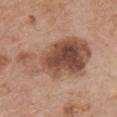| feature | finding |
|---|---|
| location | the chest |
| patient | male, aged 58 to 62 |
| diameter | ≈9.5 mm |
| automated metrics | a footprint of about 38 mm², an outline eccentricity of about 0.8 (0 = round, 1 = elongated), and a shape-asymmetry score of about 0.35 (0 = symmetric); a lesion color around L≈50 a*≈20 b*≈28 in CIELAB, a lesion–skin lightness drop of about 13, and a lesion-to-skin contrast of about 9 (normalized; higher = more distinct); an automated nevus-likeness rating near 70 out of 100 and a lesion-detection confidence of about 100/100 |
| image | total-body-photography crop, ~15 mm field of view |